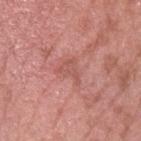workup = catalogued during a skin exam; not biopsied
tile lighting = white-light illumination
imaging modality = 15 mm crop, total-body photography
subject = male, in their 40s
size = ≈3 mm
body site = the arm